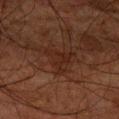biopsy status = total-body-photography surveillance lesion; no biopsy
patient = male, aged around 80
image = ~15 mm crop, total-body skin-cancer survey
lesion diameter = about 4 mm
TBP lesion metrics = a footprint of about 6.5 mm² and a symmetry-axis asymmetry near 0.6; a border-irregularity index near 6.5/10, internal color variation of about 1.5 on a 0–10 scale, and radial color variation of about 0.5
location = the left forearm
lighting = cross-polarized